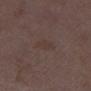Clinical impression:
The lesion was photographed on a routine skin check and not biopsied; there is no pathology result.
Image and clinical context:
Cropped from a whole-body photographic skin survey; the tile spans about 15 mm. Measured at roughly 3 mm in maximum diameter. The tile uses white-light illumination. The subject is a female aged around 35. The lesion is located on the left lower leg.